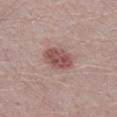Part of a total-body skin-imaging series; this lesion was reviewed on a skin check and was not flagged for biopsy. A 15 mm close-up tile from a total-body photography series done for melanoma screening. Captured under white-light illumination. An algorithmic analysis of the crop reported an area of roughly 9 mm², a shape eccentricity near 0.75, and a shape-asymmetry score of about 0.1 (0 = symmetric). The software also gave border irregularity of about 1 on a 0–10 scale, a color-variation rating of about 3.5/10, and radial color variation of about 1. Measured at roughly 4 mm in maximum diameter. The subject is a male approximately 40 years of age. On the right lower leg.